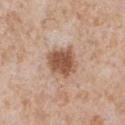Q: Was this lesion biopsied?
A: total-body-photography surveillance lesion; no biopsy
Q: Where on the body is the lesion?
A: the chest
Q: Lesion size?
A: ~3.5 mm (longest diameter)
Q: What kind of image is this?
A: ~15 mm tile from a whole-body skin photo
Q: What are the patient's age and sex?
A: male, about 65 years old